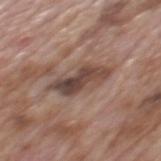workup: total-body-photography surveillance lesion; no biopsy
imaging modality: total-body-photography crop, ~15 mm field of view
lesion diameter: ~6 mm (longest diameter)
subject: male, roughly 70 years of age
location: the back
lighting: white-light illumination